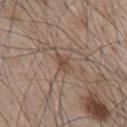| key | value |
|---|---|
| biopsy status | catalogued during a skin exam; not biopsied |
| subject | male, in their mid- to late 60s |
| lighting | white-light |
| body site | the front of the torso |
| acquisition | ~15 mm tile from a whole-body skin photo |
| diameter | ≈2.5 mm |